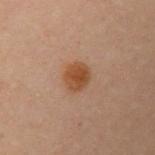Recorded during total-body skin imaging; not selected for excision or biopsy.
Cropped from a total-body skin-imaging series; the visible field is about 15 mm.
An algorithmic analysis of the crop reported an average lesion color of about L≈38 a*≈18 b*≈28 (CIELAB), roughly 8 lightness units darker than nearby skin, and a normalized border contrast of about 9. The analysis additionally found a border-irregularity index near 1.5/10, a color-variation rating of about 3/10, and radial color variation of about 1. The software also gave an automated nevus-likeness rating near 100 out of 100 and a detector confidence of about 100 out of 100 that the crop contains a lesion.
The patient is a female aged approximately 30.
The lesion is located on the left upper arm.